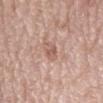Imaged during a routine full-body skin examination; the lesion was not biopsied and no histopathology is available.
Located on the mid back.
Automated image analysis of the tile measured a footprint of about 3.5 mm², an eccentricity of roughly 0.85, and a shape-asymmetry score of about 0.3 (0 = symmetric). And it measured a lesion color around L≈58 a*≈20 b*≈26 in CIELAB, roughly 9 lightness units darker than nearby skin, and a lesion-to-skin contrast of about 6 (normalized; higher = more distinct). The analysis additionally found a detector confidence of about 100 out of 100 that the crop contains a lesion.
Imaged with white-light lighting.
A male subject, aged approximately 80.
A lesion tile, about 15 mm wide, cut from a 3D total-body photograph.